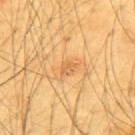Clinical impression:
Part of a total-body skin-imaging series; this lesion was reviewed on a skin check and was not flagged for biopsy.
Context:
From the upper back. The subject is a male aged 63–67. The lesion's longest dimension is about 2 mm. This is a cross-polarized tile. A close-up tile cropped from a whole-body skin photograph, about 15 mm across.